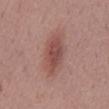<lesion>
<biopsy_status>not biopsied; imaged during a skin examination</biopsy_status>
<automated_metrics>
  <area_mm2_approx>12.0</area_mm2_approx>
  <eccentricity>0.9</eccentricity>
  <shape_asymmetry>0.2</shape_asymmetry>
</automated_metrics>
<lighting>white-light</lighting>
<lesion_size>
  <long_diameter_mm_approx>6.0</long_diameter_mm_approx>
</lesion_size>
<site>mid back</site>
<patient>
  <sex>female</sex>
  <age_approx>50</age_approx>
</patient>
<image>
  <source>total-body photography crop</source>
  <field_of_view_mm>15</field_of_view_mm>
</image>
</lesion>